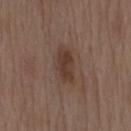Q: What are the patient's age and sex?
A: male, aged 68–72
Q: Automated lesion metrics?
A: an average lesion color of about L≈37 a*≈16 b*≈24 (CIELAB) and a normalized border contrast of about 8; border irregularity of about 2.5 on a 0–10 scale, a color-variation rating of about 2.5/10, and a peripheral color-asymmetry measure near 1
Q: How was this image acquired?
A: ~15 mm tile from a whole-body skin photo
Q: What is the anatomic site?
A: the back
Q: Illumination type?
A: white-light illumination